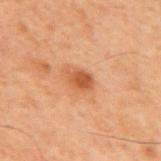Recorded during total-body skin imaging; not selected for excision or biopsy. Located on the mid back. A male patient about 70 years old. About 2.5 mm across. Captured under cross-polarized illumination. A 15 mm crop from a total-body photograph taken for skin-cancer surveillance.